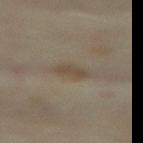notes: no biopsy performed (imaged during a skin exam)
illumination: cross-polarized illumination
image: total-body-photography crop, ~15 mm field of view
body site: the front of the torso
diameter: about 3.5 mm
patient: female, aged around 55
automated metrics: a mean CIELAB color near L≈47 a*≈9 b*≈25, a lesion–skin lightness drop of about 7, and a lesion-to-skin contrast of about 6 (normalized; higher = more distinct); a border-irregularity rating of about 3/10, internal color variation of about 1.5 on a 0–10 scale, and radial color variation of about 0.5; a classifier nevus-likeness of about 10/100 and a detector confidence of about 95 out of 100 that the crop contains a lesion